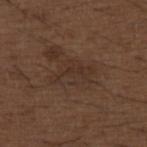No biopsy was performed on this lesion — it was imaged during a full skin examination and was not determined to be concerning. About 5 mm across. An algorithmic analysis of the crop reported an area of roughly 11 mm² and a symmetry-axis asymmetry near 0.35. It also reported a lesion–skin lightness drop of about 5. And it measured a classifier nevus-likeness of about 5/100. The lesion is located on the upper back. Imaged with white-light lighting. A male patient, about 50 years old. A 15 mm close-up tile from a total-body photography series done for melanoma screening.